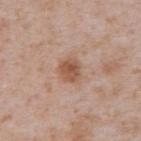Q: Is there a histopathology result?
A: catalogued during a skin exam; not biopsied
Q: Illumination type?
A: white-light illumination
Q: Patient demographics?
A: male, in their mid- to late 60s
Q: Where on the body is the lesion?
A: the abdomen
Q: What kind of image is this?
A: 15 mm crop, total-body photography